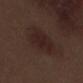Findings:
* follow-up: no biopsy performed (imaged during a skin exam)
* site: the leg
* TBP lesion metrics: a lesion area of about 15 mm² and a shape eccentricity near 0.65; radial color variation of about 1; a classifier nevus-likeness of about 40/100 and a detector confidence of about 100 out of 100 that the crop contains a lesion
* lesion diameter: ≈5 mm
* image: 15 mm crop, total-body photography
* subject: male, in their 70s